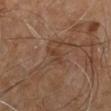Impression:
This lesion was catalogued during total-body skin photography and was not selected for biopsy.
Image and clinical context:
This is a cross-polarized tile. A lesion tile, about 15 mm wide, cut from a 3D total-body photograph. The patient is a male about 60 years old. On the lower back. The lesion-visualizer software estimated internal color variation of about 2.5 on a 0–10 scale and radial color variation of about 0.5. The software also gave a classifier nevus-likeness of about 0/100 and a detector confidence of about 65 out of 100 that the crop contains a lesion. Measured at roughly 3.5 mm in maximum diameter.